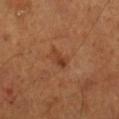workup: imaged on a skin check; not biopsied
diameter: ~2.5 mm (longest diameter)
illumination: cross-polarized
location: the right lower leg
subject: male, approximately 55 years of age
image-analysis metrics: a mean CIELAB color near L≈39 a*≈24 b*≈33, a lesion–skin lightness drop of about 7, and a normalized lesion–skin contrast near 6.5; a nevus-likeness score of about 25/100 and a detector confidence of about 100 out of 100 that the crop contains a lesion
image source: 15 mm crop, total-body photography